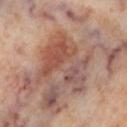| field | value |
|---|---|
| lesion size | ≈7 mm |
| acquisition | total-body-photography crop, ~15 mm field of view |
| subject | female, about 55 years old |
| illumination | cross-polarized |
| location | the left lower leg |